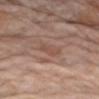| feature | finding |
|---|---|
| notes | catalogued during a skin exam; not biopsied |
| TBP lesion metrics | a mean CIELAB color near L≈50 a*≈19 b*≈25 |
| site | the arm |
| image source | ~15 mm crop, total-body skin-cancer survey |
| patient | male, aged 73–77 |